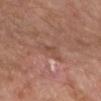Notes:
* follow-up — imaged on a skin check; not biopsied
* diameter — ≈3 mm
* image — total-body-photography crop, ~15 mm field of view
* TBP lesion metrics — an area of roughly 4.5 mm²; internal color variation of about 2.5 on a 0–10 scale; a classifier nevus-likeness of about 0/100 and a detector confidence of about 85 out of 100 that the crop contains a lesion
* anatomic site — the right forearm
* subject — about 55 years old
* illumination — cross-polarized